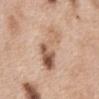Q: Was this lesion biopsied?
A: imaged on a skin check; not biopsied
Q: Lesion size?
A: ≈6.5 mm
Q: How was this image acquired?
A: ~15 mm crop, total-body skin-cancer survey
Q: What did automated image analysis measure?
A: a footprint of about 14 mm² and a shape-asymmetry score of about 0.45 (0 = symmetric); about 12 CIELAB-L* units darker than the surrounding skin and a lesion-to-skin contrast of about 7.5 (normalized; higher = more distinct)
Q: Lesion location?
A: the chest
Q: Who is the patient?
A: female, aged around 40